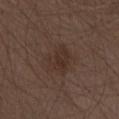<case>
  <biopsy_status>not biopsied; imaged during a skin examination</biopsy_status>
  <patient>
    <sex>male</sex>
    <age_approx>70</age_approx>
  </patient>
  <site>abdomen</site>
  <lesion_size>
    <long_diameter_mm_approx>3.5</long_diameter_mm_approx>
  </lesion_size>
  <automated_metrics>
    <border_irregularity_0_10>3.0</border_irregularity_0_10>
    <color_variation_0_10>2.5</color_variation_0_10>
    <peripheral_color_asymmetry>1.0</peripheral_color_asymmetry>
  </automated_metrics>
  <lighting>white-light</lighting>
  <image>
    <source>total-body photography crop</source>
    <field_of_view_mm>15</field_of_view_mm>
  </image>
</case>